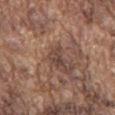Recorded during total-body skin imaging; not selected for excision or biopsy. A male subject roughly 75 years of age. On the chest. A 15 mm close-up extracted from a 3D total-body photography capture.